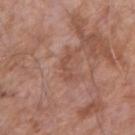Case summary:
– workup: imaged on a skin check; not biopsied
– tile lighting: white-light
– patient: male, aged 73–77
– acquisition: ~15 mm crop, total-body skin-cancer survey
– size: ~3 mm (longest diameter)
– site: the right upper arm